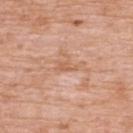This lesion was catalogued during total-body skin photography and was not selected for biopsy. The lesion is on the back. A female patient in their 70s. The lesion's longest dimension is about 2.5 mm. A 15 mm crop from a total-body photograph taken for skin-cancer surveillance. This is a white-light tile. The total-body-photography lesion software estimated a footprint of about 3 mm², a shape eccentricity near 0.85, and two-axis asymmetry of about 0.4. The software also gave a normalized border contrast of about 5.5. The analysis additionally found a border-irregularity index near 4/10, a color-variation rating of about 1/10, and peripheral color asymmetry of about 0. It also reported a detector confidence of about 100 out of 100 that the crop contains a lesion.